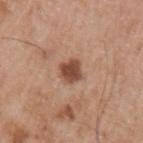Case summary:
- follow-up · catalogued during a skin exam; not biopsied
- lighting · white-light
- lesion diameter · ~2.5 mm (longest diameter)
- patient · male, roughly 55 years of age
- location · the left upper arm
- image · total-body-photography crop, ~15 mm field of view